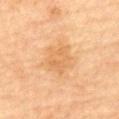Impression: Captured during whole-body skin photography for melanoma surveillance; the lesion was not biopsied. Context: The recorded lesion diameter is about 4.5 mm. Captured under cross-polarized illumination. The total-body-photography lesion software estimated an area of roughly 11 mm², a shape eccentricity near 0.55, and a shape-asymmetry score of about 0.3 (0 = symmetric). And it measured a mean CIELAB color near L≈59 a*≈19 b*≈38, roughly 6 lightness units darker than nearby skin, and a normalized border contrast of about 5. And it measured a nevus-likeness score of about 0/100 and lesion-presence confidence of about 100/100. The lesion is located on the back. A female subject aged 68–72. A 15 mm close-up tile from a total-body photography series done for melanoma screening.